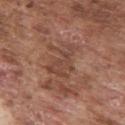Acquisition and patient details:
From the right upper arm. This image is a 15 mm lesion crop taken from a total-body photograph. Approximately 5 mm at its widest. A male subject, aged 73 to 77.